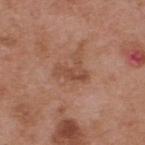Notes:
• follow-up: imaged on a skin check; not biopsied
• diameter: about 3.5 mm
• image source: ~15 mm tile from a whole-body skin photo
• patient: male, roughly 55 years of age
• site: the upper back
• lighting: white-light illumination
• TBP lesion metrics: an area of roughly 5 mm²; a within-lesion color-variation index near 2/10